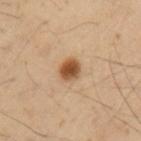<tbp_lesion>
<image>
  <source>total-body photography crop</source>
  <field_of_view_mm>15</field_of_view_mm>
</image>
<patient>
  <sex>male</sex>
  <age_approx>40</age_approx>
</patient>
<automated_metrics>
  <area_mm2_approx>5.0</area_mm2_approx>
  <eccentricity>0.6</eccentricity>
  <cielab_L>50</cielab_L>
  <cielab_a>21</cielab_a>
  <cielab_b>36</cielab_b>
  <vs_skin_darker_L>16.0</vs_skin_darker_L>
  <vs_skin_contrast_norm>11.0</vs_skin_contrast_norm>
  <border_irregularity_0_10>2.0</border_irregularity_0_10>
  <color_variation_0_10>4.0</color_variation_0_10>
  <nevus_likeness_0_100>100</nevus_likeness_0_100>
</automated_metrics>
<site>arm</site>
<lighting>cross-polarized</lighting>
<lesion_size>
  <long_diameter_mm_approx>2.5</long_diameter_mm_approx>
</lesion_size>
</tbp_lesion>